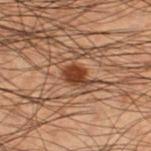Findings:
- notes · no biopsy performed (imaged during a skin exam)
- lesion size · about 2.5 mm
- location · the left thigh
- patient · male, approximately 50 years of age
- image · 15 mm crop, total-body photography